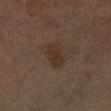- follow-up: total-body-photography surveillance lesion; no biopsy
- subject: male, about 65 years old
- automated lesion analysis: an area of roughly 5.5 mm², an eccentricity of roughly 0.9, and a shape-asymmetry score of about 0.2 (0 = symmetric); a lesion color around L≈31 a*≈13 b*≈23 in CIELAB, a lesion–skin lightness drop of about 6, and a normalized lesion–skin contrast near 6.5; border irregularity of about 2.5 on a 0–10 scale
- illumination: cross-polarized illumination
- lesion size: ≈4 mm
- imaging modality: ~15 mm crop, total-body skin-cancer survey
- site: the right lower leg